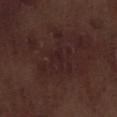This lesion was catalogued during total-body skin photography and was not selected for biopsy.
Cropped from a total-body skin-imaging series; the visible field is about 15 mm.
The lesion's longest dimension is about 6 mm.
Imaged with white-light lighting.
Automated image analysis of the tile measured a border-irregularity index near 9.5/10, internal color variation of about 2.5 on a 0–10 scale, and peripheral color asymmetry of about 0.5.
A male patient in their 70s.
The lesion is on the right lower leg.